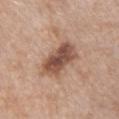{"biopsy_status": "not biopsied; imaged during a skin examination", "lesion_size": {"long_diameter_mm_approx": 5.5}, "site": "chest", "patient": {"sex": "female", "age_approx": 40}, "image": {"source": "total-body photography crop", "field_of_view_mm": 15}}